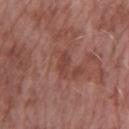No biopsy was performed on this lesion — it was imaged during a full skin examination and was not determined to be concerning. A female subject, aged around 60. An algorithmic analysis of the crop reported a mean CIELAB color near L≈43 a*≈24 b*≈25. The software also gave a border-irregularity rating of about 3.5/10, a color-variation rating of about 1/10, and peripheral color asymmetry of about 0.5. Cropped from a whole-body photographic skin survey; the tile spans about 15 mm. From the arm. About 3 mm across. The tile uses white-light illumination.